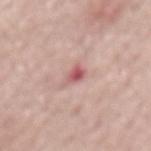Assessment:
The lesion was tiled from a total-body skin photograph and was not biopsied.
Context:
A male subject, aged 68 to 72. This image is a 15 mm lesion crop taken from a total-body photograph. The lesion is located on the mid back.